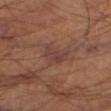The lesion was photographed on a routine skin check and not biopsied; there is no pathology result. Captured under cross-polarized illumination. A male patient roughly 70 years of age. Cropped from a whole-body photographic skin survey; the tile spans about 15 mm. The lesion is located on the right thigh.